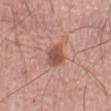biopsy status: no biopsy performed (imaged during a skin exam)
illumination: white-light illumination
size: ~3 mm (longest diameter)
image source: ~15 mm tile from a whole-body skin photo
subject: male, aged around 50
location: the abdomen